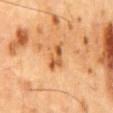body site = the mid back
image-analysis metrics = a border-irregularity rating of about 3.5/10, a within-lesion color-variation index near 7/10, and peripheral color asymmetry of about 2.5
subject = male, aged around 65
tile lighting = cross-polarized illumination
image source = ~15 mm crop, total-body skin-cancer survey